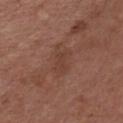Captured during whole-body skin photography for melanoma surveillance; the lesion was not biopsied. The lesion's longest dimension is about 2.5 mm. A region of skin cropped from a whole-body photographic capture, roughly 15 mm wide. The subject is a male in their mid- to late 50s. Captured under white-light illumination. From the chest.